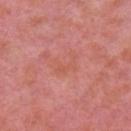Q: Is there a histopathology result?
A: catalogued during a skin exam; not biopsied
Q: What did automated image analysis measure?
A: a footprint of about 4 mm², an eccentricity of roughly 0.85, and a symmetry-axis asymmetry near 0.6; a mean CIELAB color near L≈57 a*≈30 b*≈31, roughly 4 lightness units darker than nearby skin, and a lesion-to-skin contrast of about 4.5 (normalized; higher = more distinct); an automated nevus-likeness rating near 0 out of 100 and lesion-presence confidence of about 100/100
Q: Patient demographics?
A: female, aged 38–42
Q: Lesion location?
A: the right upper arm
Q: What lighting was used for the tile?
A: white-light illumination
Q: What is the imaging modality?
A: 15 mm crop, total-body photography